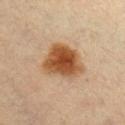* biopsy status · catalogued during a skin exam; not biopsied
* image-analysis metrics · a mean CIELAB color near L≈42 a*≈19 b*≈32 and a normalized border contrast of about 11.5
* lesion diameter · about 5 mm
* location · the chest
* subject · female, aged 48–52
* image source · ~15 mm crop, total-body skin-cancer survey